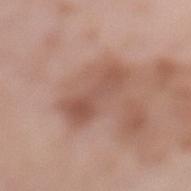Clinical impression: Captured during whole-body skin photography for melanoma surveillance; the lesion was not biopsied. Image and clinical context: Longest diameter approximately 5.5 mm. Automated image analysis of the tile measured a border-irregularity rating of about 3.5/10 and internal color variation of about 4 on a 0–10 scale. The lesion is located on the left lower leg. This is a white-light tile. A female subject in their mid- to late 60s. A 15 mm close-up extracted from a 3D total-body photography capture.